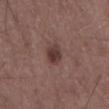The lesion was tiled from a total-body skin photograph and was not biopsied.